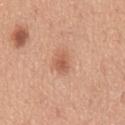Q: Is there a histopathology result?
A: catalogued during a skin exam; not biopsied
Q: Automated lesion metrics?
A: an area of roughly 4 mm², a shape eccentricity near 0.75, and two-axis asymmetry of about 0.3; lesion-presence confidence of about 100/100
Q: Patient demographics?
A: male, approximately 50 years of age
Q: Illumination type?
A: white-light
Q: How large is the lesion?
A: about 3 mm
Q: Lesion location?
A: the mid back
Q: How was this image acquired?
A: 15 mm crop, total-body photography